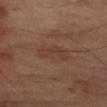biopsy status: catalogued during a skin exam; not biopsied
subject: male, aged approximately 70
acquisition: ~15 mm tile from a whole-body skin photo
body site: the leg